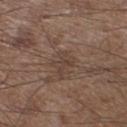follow-up: no biopsy performed (imaged during a skin exam)
lighting: white-light
acquisition: total-body-photography crop, ~15 mm field of view
size: about 3.5 mm
location: the left lower leg
patient: male, about 55 years old